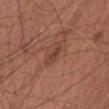  biopsy_status: not biopsied; imaged during a skin examination
  patient:
    sex: female
    age_approx: 65
  lighting: white-light
  site: head or neck
  image:
    source: total-body photography crop
    field_of_view_mm: 15